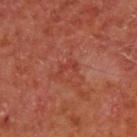The lesion is located on the upper back. About 2.5 mm across. A male patient, about 65 years old. This image is a 15 mm lesion crop taken from a total-body photograph. This is a cross-polarized tile. Automated tile analysis of the lesion measured an area of roughly 2.5 mm², an eccentricity of roughly 0.9, and two-axis asymmetry of about 0.65.